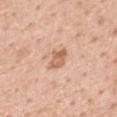Recorded during total-body skin imaging; not selected for excision or biopsy. The lesion is on the mid back. A male patient aged approximately 60. A 15 mm close-up extracted from a 3D total-body photography capture. This is a white-light tile. Measured at roughly 2.5 mm in maximum diameter.